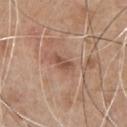Recorded during total-body skin imaging; not selected for excision or biopsy. About 3 mm across. The tile uses white-light illumination. A region of skin cropped from a whole-body photographic capture, roughly 15 mm wide. A male patient, approximately 65 years of age. From the chest.